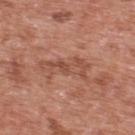Assessment:
Recorded during total-body skin imaging; not selected for excision or biopsy.
Acquisition and patient details:
A male patient aged 68 to 72. This image is a 15 mm lesion crop taken from a total-body photograph. On the upper back.